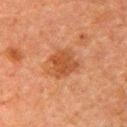From the right upper arm. A male patient, aged approximately 60. Cropped from a total-body skin-imaging series; the visible field is about 15 mm. The tile uses cross-polarized illumination. Measured at roughly 4 mm in maximum diameter.